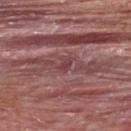This lesion was catalogued during total-body skin photography and was not selected for biopsy.
On the upper back.
The patient is a male about 50 years old.
A 15 mm close-up tile from a total-body photography series done for melanoma screening.